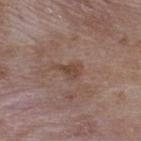{
  "biopsy_status": "not biopsied; imaged during a skin examination",
  "image": {
    "source": "total-body photography crop",
    "field_of_view_mm": 15
  },
  "site": "upper back",
  "lesion_size": {
    "long_diameter_mm_approx": 3.0
  },
  "lighting": "white-light",
  "patient": {
    "sex": "male",
    "age_approx": 50
  }
}